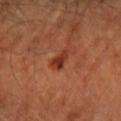Notes:
- notes · no biopsy performed (imaged during a skin exam)